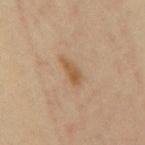Captured during whole-body skin photography for melanoma surveillance; the lesion was not biopsied. The lesion is on the back. The subject is a female roughly 45 years of age. A 15 mm close-up extracted from a 3D total-body photography capture.